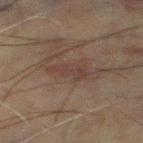Q: Was a biopsy performed?
A: imaged on a skin check; not biopsied
Q: Where on the body is the lesion?
A: the left thigh
Q: What is the lesion's diameter?
A: about 4 mm
Q: What is the imaging modality?
A: ~15 mm crop, total-body skin-cancer survey
Q: Illumination type?
A: cross-polarized
Q: Patient demographics?
A: male, roughly 60 years of age